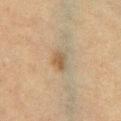A female patient about 55 years old.
Measured at roughly 2.5 mm in maximum diameter.
Imaged with cross-polarized lighting.
The lesion is located on the front of the torso.
A region of skin cropped from a whole-body photographic capture, roughly 15 mm wide.